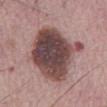<lesion>
  <biopsy_status>not biopsied; imaged during a skin examination</biopsy_status>
</lesion>